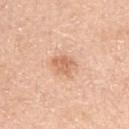No biopsy was performed on this lesion — it was imaged during a full skin examination and was not determined to be concerning. A region of skin cropped from a whole-body photographic capture, roughly 15 mm wide. Located on the left upper arm. The recorded lesion diameter is about 3 mm. Captured under white-light illumination. A male patient aged 63–67.